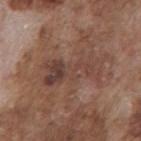biopsy_status: not biopsied; imaged during a skin examination
automated_metrics:
  area_mm2_approx: 15.0
  eccentricity: 0.9
  shape_asymmetry: 0.4
  border_irregularity_0_10: 7.5
  color_variation_0_10: 6.0
  peripheral_color_asymmetry: 1.5
  lesion_detection_confidence_0_100: 95
patient:
  sex: male
  age_approx: 75
site: front of the torso
image:
  source: total-body photography crop
  field_of_view_mm: 15
lesion_size:
  long_diameter_mm_approx: 7.0